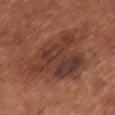Imaged during a routine full-body skin examination; the lesion was not biopsied and no histopathology is available. A female patient in their mid- to late 60s. The lesion-visualizer software estimated two-axis asymmetry of about 0.45. And it measured an average lesion color of about L≈39 a*≈23 b*≈26 (CIELAB), roughly 9 lightness units darker than nearby skin, and a normalized lesion–skin contrast near 8. The software also gave a border-irregularity rating of about 6/10. Cropped from a whole-body photographic skin survey; the tile spans about 15 mm. From the chest. Approximately 11 mm at its widest.